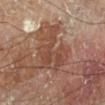biopsy status: no biopsy performed (imaged during a skin exam)
lesion size: about 6 mm
subject: male, in their 70s
automated lesion analysis: a border-irregularity index near 7/10, internal color variation of about 4 on a 0–10 scale, and peripheral color asymmetry of about 1.5; a classifier nevus-likeness of about 0/100 and lesion-presence confidence of about 95/100
acquisition: 15 mm crop, total-body photography
body site: the leg